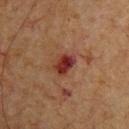workup: no biopsy performed (imaged during a skin exam) | anatomic site: the chest | size: ~3 mm (longest diameter) | image source: 15 mm crop, total-body photography | patient: male, aged 63 to 67.